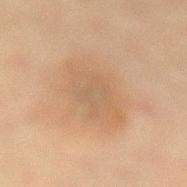A female patient approximately 50 years of age. Captured under cross-polarized illumination. The lesion is located on the lower back. A 15 mm close-up extracted from a 3D total-body photography capture. Approximately 7.5 mm at its widest.